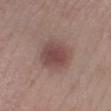{
  "biopsy_status": "not biopsied; imaged during a skin examination",
  "image": {
    "source": "total-body photography crop",
    "field_of_view_mm": 15
  },
  "site": "right lower leg",
  "patient": {
    "sex": "male",
    "age_approx": 20
  },
  "lesion_size": {
    "long_diameter_mm_approx": 4.0
  },
  "lighting": "white-light"
}